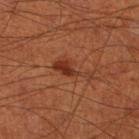The lesion was photographed on a routine skin check and not biopsied; there is no pathology result.
Cropped from a total-body skin-imaging series; the visible field is about 15 mm.
On the right thigh.
Automated image analysis of the tile measured an average lesion color of about L≈34 a*≈26 b*≈31 (CIELAB) and roughly 9 lightness units darker than nearby skin. And it measured a border-irregularity rating of about 7/10, a within-lesion color-variation index near 2.5/10, and a peripheral color-asymmetry measure near 1. The software also gave a nevus-likeness score of about 90/100 and lesion-presence confidence of about 100/100.
The tile uses cross-polarized illumination.
A male patient, aged around 70.